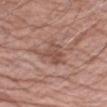The lesion was tiled from a total-body skin photograph and was not biopsied. This image is a 15 mm lesion crop taken from a total-body photograph. On the left upper arm. A male patient aged around 75.